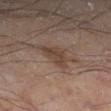The lesion was tiled from a total-body skin photograph and was not biopsied. A male subject, in their mid-50s. Automated tile analysis of the lesion measured a lesion area of about 7 mm², an eccentricity of roughly 0.7, and two-axis asymmetry of about 0.45. And it measured a lesion–skin lightness drop of about 7 and a normalized lesion–skin contrast near 7. It also reported internal color variation of about 3 on a 0–10 scale and radial color variation of about 1. The lesion is on the leg. A 15 mm close-up tile from a total-body photography series done for melanoma screening. Imaged with cross-polarized lighting. The recorded lesion diameter is about 4 mm.